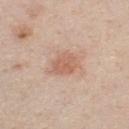Located on the chest. The subject is a male about 45 years old. A 15 mm close-up extracted from a 3D total-body photography capture.